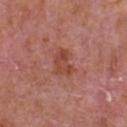Impression: The lesion was tiled from a total-body skin photograph and was not biopsied. Image and clinical context: A male subject aged 63–67. Approximately 3.5 mm at its widest. On the chest. This image is a 15 mm lesion crop taken from a total-body photograph. Captured under white-light illumination.